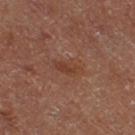  biopsy_status: not biopsied; imaged during a skin examination
  automated_metrics:
    cielab_L: 41
    cielab_a: 22
    cielab_b: 29
    vs_skin_darker_L: 6.0
    vs_skin_contrast_norm: 6.0
    color_variation_0_10: 3.0
    peripheral_color_asymmetry: 1.0
  patient:
    sex: female
  image:
    source: total-body photography crop
    field_of_view_mm: 15
  site: right thigh
  lighting: cross-polarized